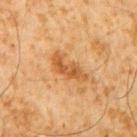The lesion was tiled from a total-body skin photograph and was not biopsied.
A male subject aged approximately 60.
On the right upper arm.
A 15 mm crop from a total-body photograph taken for skin-cancer surveillance.